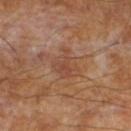Assessment:
The lesion was tiled from a total-body skin photograph and was not biopsied.
Clinical summary:
A region of skin cropped from a whole-body photographic capture, roughly 15 mm wide. Longest diameter approximately 3.5 mm. Automated image analysis of the tile measured a border-irregularity rating of about 4/10 and radial color variation of about 1. A male patient aged around 65. This is a cross-polarized tile.